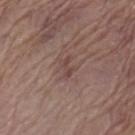{"biopsy_status": "not biopsied; imaged during a skin examination", "site": "left thigh", "lesion_size": {"long_diameter_mm_approx": 2.5}, "patient": {"sex": "female", "age_approx": 70}, "lighting": "white-light", "automated_metrics": {"border_irregularity_0_10": 6.5, "color_variation_0_10": 0.0, "peripheral_color_asymmetry": 0.0}, "image": {"source": "total-body photography crop", "field_of_view_mm": 15}}